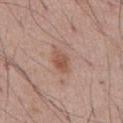Context:
A male patient, roughly 55 years of age. On the abdomen. A lesion tile, about 15 mm wide, cut from a 3D total-body photograph. The tile uses white-light illumination. The lesion-visualizer software estimated an eccentricity of roughly 0.8 and two-axis asymmetry of about 0.25. The analysis additionally found a nevus-likeness score of about 80/100 and a detector confidence of about 100 out of 100 that the crop contains a lesion.